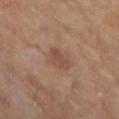{"biopsy_status": "not biopsied; imaged during a skin examination", "lighting": "cross-polarized", "patient": {"sex": "female", "age_approx": 50}, "automated_metrics": {"cielab_L": 46, "cielab_a": 19, "cielab_b": 27, "vs_skin_darker_L": 7.0, "vs_skin_contrast_norm": 5.5, "nevus_likeness_0_100": 25}, "site": "left thigh", "lesion_size": {"long_diameter_mm_approx": 3.0}, "image": {"source": "total-body photography crop", "field_of_view_mm": 15}}